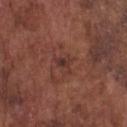Clinical impression:
Part of a total-body skin-imaging series; this lesion was reviewed on a skin check and was not flagged for biopsy.
Clinical summary:
The lesion is on the chest. An algorithmic analysis of the crop reported an average lesion color of about L≈34 a*≈21 b*≈22 (CIELAB). The patient is a male approximately 75 years of age. A 15 mm close-up extracted from a 3D total-body photography capture. Captured under white-light illumination. Longest diameter approximately 2.5 mm.